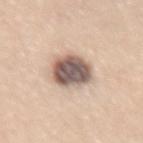Q: Is there a histopathology result?
A: total-body-photography surveillance lesion; no biopsy
Q: Patient demographics?
A: female, in their mid-60s
Q: What kind of image is this?
A: 15 mm crop, total-body photography
Q: Where on the body is the lesion?
A: the lower back